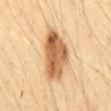Clinical impression:
The lesion was photographed on a routine skin check and not biopsied; there is no pathology result.